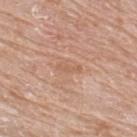follow-up = catalogued during a skin exam; not biopsied | subject = male, aged 63–67 | image = 15 mm crop, total-body photography | anatomic site = the upper back.